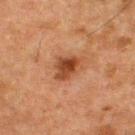{
  "biopsy_status": "not biopsied; imaged during a skin examination",
  "lighting": "cross-polarized",
  "patient": {
    "sex": "male",
    "age_approx": 50
  },
  "image": {
    "source": "total-body photography crop",
    "field_of_view_mm": 15
  },
  "site": "upper back"
}